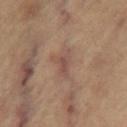Findings:
– acquisition: ~15 mm tile from a whole-body skin photo
– lesion size: ~3.5 mm (longest diameter)
– automated lesion analysis: a footprint of about 5.5 mm², a shape eccentricity near 0.8, and a symmetry-axis asymmetry near 0.5
– patient: female, roughly 65 years of age
– tile lighting: cross-polarized
– body site: the right thigh